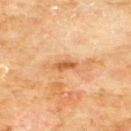Impression:
This lesion was catalogued during total-body skin photography and was not selected for biopsy.
Clinical summary:
A roughly 15 mm field-of-view crop from a total-body skin photograph. A male subject aged around 70. Imaged with cross-polarized lighting. The total-body-photography lesion software estimated an area of roughly 3 mm², a shape eccentricity near 0.85, and two-axis asymmetry of about 0.3. It also reported an average lesion color of about L≈49 a*≈21 b*≈37 (CIELAB), about 10 CIELAB-L* units darker than the surrounding skin, and a lesion-to-skin contrast of about 7.5 (normalized; higher = more distinct). It also reported a within-lesion color-variation index near 1/10 and a peripheral color-asymmetry measure near 0.5. And it measured an automated nevus-likeness rating near 0 out of 100 and a lesion-detection confidence of about 100/100. The lesion is located on the back. Approximately 2.5 mm at its widest.